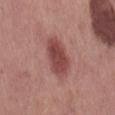Clinical impression:
No biopsy was performed on this lesion — it was imaged during a full skin examination and was not determined to be concerning.
Context:
A male subject, aged 58 to 62. Cropped from a whole-body photographic skin survey; the tile spans about 15 mm. The lesion is on the back.